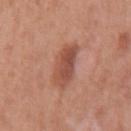Q: What kind of image is this?
A: total-body-photography crop, ~15 mm field of view
Q: What are the patient's age and sex?
A: male, aged approximately 70
Q: Lesion location?
A: the back